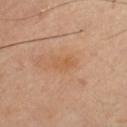Clinical impression: The lesion was photographed on a routine skin check and not biopsied; there is no pathology result. Background: Captured under cross-polarized illumination. A male patient, aged 38–42. From the chest. Cropped from a total-body skin-imaging series; the visible field is about 15 mm.